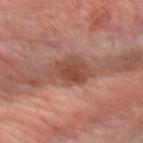The lesion was tiled from a total-body skin photograph and was not biopsied. Cropped from a whole-body photographic skin survey; the tile spans about 15 mm. Located on the left forearm. About 5 mm across. A female patient aged approximately 65.